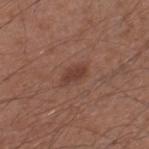The lesion is on the right lower leg.
An algorithmic analysis of the crop reported an area of roughly 4 mm², a shape eccentricity near 0.85, and a symmetry-axis asymmetry near 0.3. It also reported roughly 8 lightness units darker than nearby skin and a normalized lesion–skin contrast near 7. The software also gave a color-variation rating of about 1/10 and a peripheral color-asymmetry measure near 0.5.
A 15 mm close-up extracted from a 3D total-body photography capture.
The recorded lesion diameter is about 3 mm.
The subject is a male aged 53 to 57.